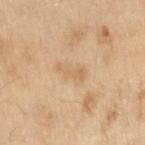Assessment: Recorded during total-body skin imaging; not selected for excision or biopsy. Background: The lesion's longest dimension is about 3.5 mm. The subject is a male roughly 70 years of age. The lesion is located on the right upper arm. A 15 mm close-up extracted from a 3D total-body photography capture.